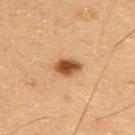Q: Was this lesion biopsied?
A: catalogued during a skin exam; not biopsied
Q: Who is the patient?
A: male, aged 58 to 62
Q: How was this image acquired?
A: ~15 mm crop, total-body skin-cancer survey